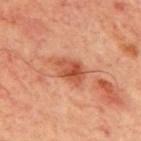Assessment: Imaged during a routine full-body skin examination; the lesion was not biopsied and no histopathology is available. Background: On the mid back. An algorithmic analysis of the crop reported a lesion area of about 8 mm², a shape eccentricity near 0.75, and two-axis asymmetry of about 0.2. The analysis additionally found border irregularity of about 2.5 on a 0–10 scale and a within-lesion color-variation index near 6.5/10. And it measured an automated nevus-likeness rating near 25 out of 100 and a lesion-detection confidence of about 100/100. The subject is a male in their 70s. Imaged with cross-polarized lighting. A 15 mm close-up extracted from a 3D total-body photography capture. The recorded lesion diameter is about 4 mm.